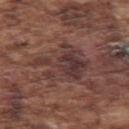Assessment: Part of a total-body skin-imaging series; this lesion was reviewed on a skin check and was not flagged for biopsy. Clinical summary: An algorithmic analysis of the crop reported a footprint of about 18 mm² and a symmetry-axis asymmetry near 0.5. It also reported a lesion color around L≈36 a*≈19 b*≈19 in CIELAB, roughly 8 lightness units darker than nearby skin, and a lesion-to-skin contrast of about 8 (normalized; higher = more distinct). And it measured a border-irregularity index near 7.5/10 and a peripheral color-asymmetry measure near 2. From the left upper arm. Cropped from a total-body skin-imaging series; the visible field is about 15 mm. About 6.5 mm across. A male patient in their mid- to late 70s.